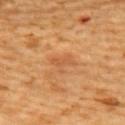Assessment:
The lesion was photographed on a routine skin check and not biopsied; there is no pathology result.
Acquisition and patient details:
A female patient roughly 65 years of age. On the upper back. Automated tile analysis of the lesion measured a footprint of about 3.5 mm², an eccentricity of roughly 0.75, and a shape-asymmetry score of about 0.5 (0 = symmetric). It also reported border irregularity of about 6 on a 0–10 scale, a within-lesion color-variation index near 1.5/10, and radial color variation of about 0.5. A 15 mm close-up extracted from a 3D total-body photography capture.